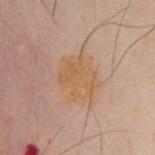| field | value |
|---|---|
| notes | total-body-photography surveillance lesion; no biopsy |
| diameter | about 4.5 mm |
| lighting | cross-polarized illumination |
| image source | total-body-photography crop, ~15 mm field of view |
| subject | male, aged approximately 50 |
| body site | the chest |